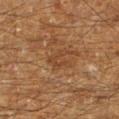The lesion was tiled from a total-body skin photograph and was not biopsied.
Automated image analysis of the tile measured a mean CIELAB color near L≈32 a*≈17 b*≈27 and a lesion–skin lightness drop of about 5. The software also gave a detector confidence of about 65 out of 100 that the crop contains a lesion.
The lesion is located on the left lower leg.
Cropped from a whole-body photographic skin survey; the tile spans about 15 mm.
This is a cross-polarized tile.
A male subject aged approximately 60.